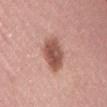Assessment: No biopsy was performed on this lesion — it was imaged during a full skin examination and was not determined to be concerning. Clinical summary: The tile uses white-light illumination. This image is a 15 mm lesion crop taken from a total-body photograph. A female subject in their mid-50s. The lesion is on the lower back. About 4.5 mm across. Automated image analysis of the tile measured a lesion area of about 10 mm², a shape eccentricity near 0.75, and a shape-asymmetry score of about 0.15 (0 = symmetric). And it measured an automated nevus-likeness rating near 95 out of 100 and a lesion-detection confidence of about 100/100.